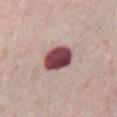No biopsy was performed on this lesion — it was imaged during a full skin examination and was not determined to be concerning. Located on the abdomen. A region of skin cropped from a whole-body photographic capture, roughly 15 mm wide. A male subject, in their mid- to late 70s.